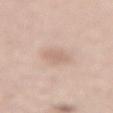  biopsy_status: not biopsied; imaged during a skin examination
  image:
    source: total-body photography crop
    field_of_view_mm: 15
  site: lower back
  patient:
    sex: male
    age_approx: 65
  automated_metrics:
    cielab_L: 66
    cielab_a: 16
    cielab_b: 26
    vs_skin_darker_L: 8.0
    nevus_likeness_0_100: 0
    lesion_detection_confidence_0_100: 100
  lesion_size:
    long_diameter_mm_approx: 3.0
  lighting: white-light